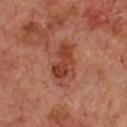Impression: This lesion was catalogued during total-body skin photography and was not selected for biopsy. Context: The lesion is located on the chest. About 5.5 mm across. A lesion tile, about 15 mm wide, cut from a 3D total-body photograph. Automated image analysis of the tile measured border irregularity of about 4.5 on a 0–10 scale and a color-variation rating of about 4/10. And it measured a classifier nevus-likeness of about 5/100 and a detector confidence of about 100 out of 100 that the crop contains a lesion. Captured under cross-polarized illumination. A male subject, aged 68 to 72.